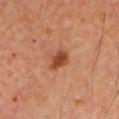Findings:
* size: ~3 mm (longest diameter)
* location: the left upper arm
* image source: total-body-photography crop, ~15 mm field of view
* TBP lesion metrics: a lesion area of about 4.5 mm², a shape eccentricity near 0.75, and two-axis asymmetry of about 0.25; a lesion color around L≈46 a*≈29 b*≈36 in CIELAB, roughly 13 lightness units darker than nearby skin, and a normalized border contrast of about 9.5
* subject: male, in their mid- to late 60s